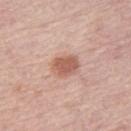<record>
<biopsy_status>not biopsied; imaged during a skin examination</biopsy_status>
<lighting>white-light</lighting>
<patient>
  <sex>female</sex>
  <age_approx>65</age_approx>
</patient>
<site>right thigh</site>
<image>
  <source>total-body photography crop</source>
  <field_of_view_mm>15</field_of_view_mm>
</image>
<lesion_size>
  <long_diameter_mm_approx>3.0</long_diameter_mm_approx>
</lesion_size>
</record>